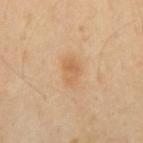* notes · no biopsy performed (imaged during a skin exam)
* lighting · cross-polarized
* automated lesion analysis · a footprint of about 5.5 mm², an outline eccentricity of about 0.8 (0 = round, 1 = elongated), and a shape-asymmetry score of about 0.2 (0 = symmetric); a lesion color around L≈60 a*≈19 b*≈37 in CIELAB
* site · the mid back
* patient · male, aged 53–57
* imaging modality · total-body-photography crop, ~15 mm field of view